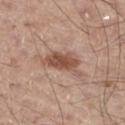Findings:
* workup · total-body-photography surveillance lesion; no biopsy
* subject · male, aged approximately 60
* lesion diameter · ~5 mm (longest diameter)
* location · the right thigh
* illumination · white-light
* acquisition · 15 mm crop, total-body photography